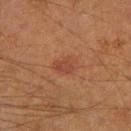Assessment: Part of a total-body skin-imaging series; this lesion was reviewed on a skin check and was not flagged for biopsy.